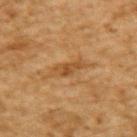Notes:
– workup: no biopsy performed (imaged during a skin exam)
– body site: the upper back
– patient: male, about 85 years old or older
– image: ~15 mm tile from a whole-body skin photo
– lesion size: ≈3 mm